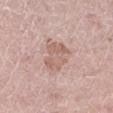| field | value |
|---|---|
| workup | catalogued during a skin exam; not biopsied |
| lesion diameter | about 4.5 mm |
| automated lesion analysis | border irregularity of about 4 on a 0–10 scale and peripheral color asymmetry of about 1 |
| body site | the leg |
| patient | male, about 75 years old |
| tile lighting | white-light illumination |
| acquisition | ~15 mm crop, total-body skin-cancer survey |